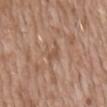The lesion was photographed on a routine skin check and not biopsied; there is no pathology result. From the abdomen. The subject is a male aged around 60. A region of skin cropped from a whole-body photographic capture, roughly 15 mm wide. Approximately 2.5 mm at its widest. Imaged with white-light lighting.